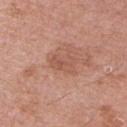This is a white-light tile.
A 15 mm close-up tile from a total-body photography series done for melanoma screening.
A male subject, aged approximately 50.
The recorded lesion diameter is about 3 mm.
The lesion is located on the left upper arm.
Automated image analysis of the tile measured a normalized lesion–skin contrast near 5. The analysis additionally found an automated nevus-likeness rating near 0 out of 100.